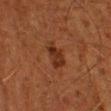  biopsy_status: not biopsied; imaged during a skin examination